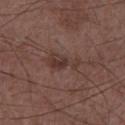biopsy_status: not biopsied; imaged during a skin examination
lighting: white-light
site: chest
image:
  source: total-body photography crop
  field_of_view_mm: 15
patient:
  sex: male
  age_approx: 60